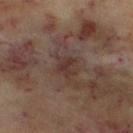follow-up = catalogued during a skin exam; not biopsied
image = total-body-photography crop, ~15 mm field of view
automated lesion analysis = a mean CIELAB color near L≈31 a*≈16 b*≈19, about 6 CIELAB-L* units darker than the surrounding skin, and a normalized lesion–skin contrast near 6.5; a border-irregularity rating of about 4/10, a within-lesion color-variation index near 3.5/10, and peripheral color asymmetry of about 1; a classifier nevus-likeness of about 0/100 and a detector confidence of about 100 out of 100 that the crop contains a lesion
anatomic site = the leg
tile lighting = cross-polarized
patient = female, in their 60s